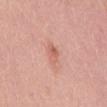{"biopsy_status": "not biopsied; imaged during a skin examination", "patient": {"sex": "female", "age_approx": 55}, "image": {"source": "total-body photography crop", "field_of_view_mm": 15}, "site": "mid back", "lighting": "white-light", "lesion_size": {"long_diameter_mm_approx": 2.5}, "automated_metrics": {"area_mm2_approx": 3.0, "shape_asymmetry": 0.25, "vs_skin_darker_L": 8.0, "vs_skin_contrast_norm": 6.0, "color_variation_0_10": 2.5, "peripheral_color_asymmetry": 1.0}}